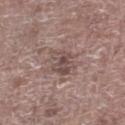| key | value |
|---|---|
| image | ~15 mm crop, total-body skin-cancer survey |
| lesion size | ~4 mm (longest diameter) |
| anatomic site | the right lower leg |
| patient | male, about 70 years old |